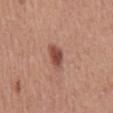Impression:
Recorded during total-body skin imaging; not selected for excision or biopsy.